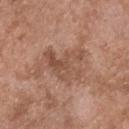Assessment:
The lesion was tiled from a total-body skin photograph and was not biopsied.
Acquisition and patient details:
Captured under white-light illumination. A 15 mm close-up extracted from a 3D total-body photography capture. The patient is a male about 50 years old. On the head or neck.